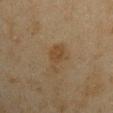The lesion was photographed on a routine skin check and not biopsied; there is no pathology result. A 15 mm close-up extracted from a 3D total-body photography capture. Measured at roughly 4 mm in maximum diameter. The subject is a male approximately 45 years of age. This is a cross-polarized tile. The lesion is on the left upper arm.